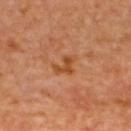Imaged during a routine full-body skin examination; the lesion was not biopsied and no histopathology is available. A 15 mm close-up tile from a total-body photography series done for melanoma screening. From the upper back. The lesion's longest dimension is about 2.5 mm. This is a cross-polarized tile. A male patient, aged around 60.